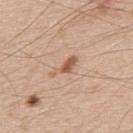biopsy status: total-body-photography surveillance lesion; no biopsy | image source: total-body-photography crop, ~15 mm field of view | automated metrics: a nevus-likeness score of about 60/100 and a lesion-detection confidence of about 100/100 | anatomic site: the upper back | subject: male, aged approximately 50 | tile lighting: white-light.